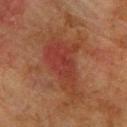  biopsy_status: not biopsied; imaged during a skin examination
  site: upper back
  image:
    source: total-body photography crop
    field_of_view_mm: 15
  patient:
    sex: male
    age_approx: 75
  lighting: cross-polarized
  lesion_size:
    long_diameter_mm_approx: 8.0
  automated_metrics:
    area_mm2_approx: 22.0
    shape_asymmetry: 0.4
    cielab_L: 32
    cielab_a: 24
    cielab_b: 26
    vs_skin_darker_L: 7.0